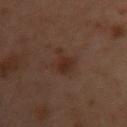{
  "biopsy_status": "not biopsied; imaged during a skin examination"
}